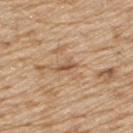workup: catalogued during a skin exam; not biopsied | subject: male, aged 68 to 72 | imaging modality: ~15 mm tile from a whole-body skin photo | automated metrics: an area of roughly 2.5 mm²; about 10 CIELAB-L* units darker than the surrounding skin and a lesion-to-skin contrast of about 6.5 (normalized; higher = more distinct) | lighting: white-light | site: the upper back.